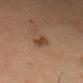Cropped from a total-body skin-imaging series; the visible field is about 15 mm.
Captured under cross-polarized illumination.
A male subject, approximately 65 years of age.